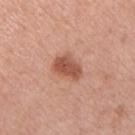Imaged during a routine full-body skin examination; the lesion was not biopsied and no histopathology is available. A male subject about 60 years old. Cropped from a total-body skin-imaging series; the visible field is about 15 mm. Approximately 3.5 mm at its widest. The total-body-photography lesion software estimated a lesion color around L≈53 a*≈25 b*≈31 in CIELAB, a lesion–skin lightness drop of about 12, and a normalized lesion–skin contrast near 8.5. And it measured a border-irregularity index near 2.5/10, internal color variation of about 3 on a 0–10 scale, and radial color variation of about 1. And it measured a nevus-likeness score of about 95/100 and a lesion-detection confidence of about 100/100. From the chest.